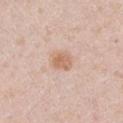  lesion_size:
    long_diameter_mm_approx: 2.5
  image:
    source: total-body photography crop
    field_of_view_mm: 15
  lighting: white-light
  patient:
    sex: male
    age_approx: 35
  site: right upper arm